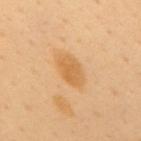Impression:
Captured during whole-body skin photography for melanoma surveillance; the lesion was not biopsied.